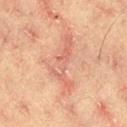{"biopsy_status": "not biopsied; imaged during a skin examination", "patient": {"sex": "male", "age_approx": 65}, "site": "right thigh", "image": {"source": "total-body photography crop", "field_of_view_mm": 15}}